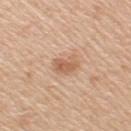A female patient, aged 48 to 52.
The lesion is on the arm.
The lesion's longest dimension is about 3 mm.
A roughly 15 mm field-of-view crop from a total-body skin photograph.
The total-body-photography lesion software estimated an area of roughly 5.5 mm², a shape eccentricity near 0.8, and a shape-asymmetry score of about 0.3 (0 = symmetric). The software also gave an average lesion color of about L≈61 a*≈21 b*≈33 (CIELAB) and about 10 CIELAB-L* units darker than the surrounding skin. The software also gave border irregularity of about 3 on a 0–10 scale, a within-lesion color-variation index near 2.5/10, and radial color variation of about 1. The analysis additionally found a lesion-detection confidence of about 100/100.
Imaged with white-light lighting.